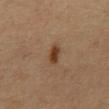follow-up = imaged on a skin check; not biopsied | lesion diameter = about 3 mm | imaging modality = total-body-photography crop, ~15 mm field of view | anatomic site = the mid back | lighting = cross-polarized | patient = male, roughly 60 years of age.